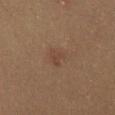Clinical impression:
This lesion was catalogued during total-body skin photography and was not selected for biopsy.
Background:
Automated tile analysis of the lesion measured a lesion area of about 4 mm², an outline eccentricity of about 0.7 (0 = round, 1 = elongated), and a shape-asymmetry score of about 0.4 (0 = symmetric). It also reported a mean CIELAB color near L≈35 a*≈15 b*≈22, a lesion–skin lightness drop of about 5, and a normalized lesion–skin contrast near 4.5. The software also gave a border-irregularity rating of about 4/10, a within-lesion color-variation index near 1.5/10, and radial color variation of about 0.5. The patient is a female in their 50s. Located on the right thigh. Measured at roughly 3 mm in maximum diameter. A roughly 15 mm field-of-view crop from a total-body skin photograph. This is a cross-polarized tile.